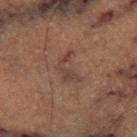Background:
Automated tile analysis of the lesion measured border irregularity of about 5.5 on a 0–10 scale, a color-variation rating of about 3/10, and peripheral color asymmetry of about 1. The lesion's longest dimension is about 4 mm. From the right thigh. This is a cross-polarized tile. A region of skin cropped from a whole-body photographic capture, roughly 15 mm wide. A male patient, aged 73 to 77.